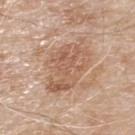Q: Was this lesion biopsied?
A: imaged on a skin check; not biopsied
Q: What kind of image is this?
A: 15 mm crop, total-body photography
Q: Patient demographics?
A: male, aged 78–82
Q: Automated lesion metrics?
A: a lesion color around L≈58 a*≈19 b*≈31 in CIELAB and a lesion-to-skin contrast of about 6 (normalized; higher = more distinct)
Q: What lighting was used for the tile?
A: white-light
Q: How large is the lesion?
A: ≈6.5 mm
Q: Where on the body is the lesion?
A: the upper back